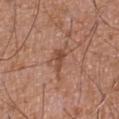Part of a total-body skin-imaging series; this lesion was reviewed on a skin check and was not flagged for biopsy. The lesion is on the abdomen. The total-body-photography lesion software estimated a lesion area of about 3.5 mm², an eccentricity of roughly 0.85, and a shape-asymmetry score of about 0.35 (0 = symmetric). The analysis additionally found a lesion–skin lightness drop of about 9 and a normalized lesion–skin contrast near 7. The software also gave a classifier nevus-likeness of about 20/100. A male patient aged around 75. A lesion tile, about 15 mm wide, cut from a 3D total-body photograph.